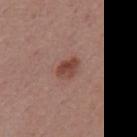Clinical impression:
The lesion was photographed on a routine skin check and not biopsied; there is no pathology result.
Image and clinical context:
This is a white-light tile. Measured at roughly 3 mm in maximum diameter. Cropped from a whole-body photographic skin survey; the tile spans about 15 mm. The subject is a male in their mid-50s. An algorithmic analysis of the crop reported a footprint of about 5 mm² and two-axis asymmetry of about 0.2. The software also gave about 10 CIELAB-L* units darker than the surrounding skin. On the mid back.